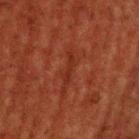Notes:
- biopsy status · catalogued during a skin exam; not biopsied
- anatomic site · the back
- image · ~15 mm crop, total-body skin-cancer survey
- subject · male, about 60 years old